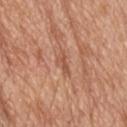Captured during whole-body skin photography for melanoma surveillance; the lesion was not biopsied. This is a white-light tile. A 15 mm close-up extracted from a 3D total-body photography capture. Longest diameter approximately 3 mm. A male patient about 80 years old. From the head or neck.